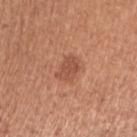Q: Is there a histopathology result?
A: catalogued during a skin exam; not biopsied
Q: What lighting was used for the tile?
A: white-light
Q: How was this image acquired?
A: 15 mm crop, total-body photography
Q: Patient demographics?
A: female, approximately 50 years of age
Q: Lesion size?
A: ≈3 mm
Q: What is the anatomic site?
A: the arm